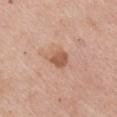imaging modality = ~15 mm crop, total-body skin-cancer survey; subject = male, aged around 55; body site = the right upper arm; diameter = ≈3 mm.